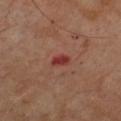Q: Was a biopsy performed?
A: imaged on a skin check; not biopsied